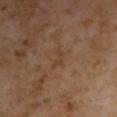Impression: No biopsy was performed on this lesion — it was imaged during a full skin examination and was not determined to be concerning. Acquisition and patient details: Captured under cross-polarized illumination. Automated tile analysis of the lesion measured a border-irregularity index near 6/10 and internal color variation of about 0 on a 0–10 scale. It also reported a lesion-detection confidence of about 100/100. A male subject, roughly 55 years of age. This image is a 15 mm lesion crop taken from a total-body photograph. The lesion is located on the left upper arm. Measured at roughly 3 mm in maximum diameter.